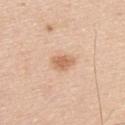follow-up: catalogued during a skin exam; not biopsied | tile lighting: white-light | patient: male, about 45 years old | imaging modality: 15 mm crop, total-body photography | anatomic site: the upper back | lesion size: ~3 mm (longest diameter).